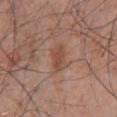Imaged during a routine full-body skin examination; the lesion was not biopsied and no histopathology is available. Cropped from a total-body skin-imaging series; the visible field is about 15 mm. This is a white-light tile. A male subject, approximately 70 years of age. Automated image analysis of the tile measured a lesion area of about 6 mm² and a symmetry-axis asymmetry near 0.25. And it measured a lesion color around L≈49 a*≈21 b*≈28 in CIELAB and roughly 7 lightness units darker than nearby skin. And it measured a color-variation rating of about 2.5/10 and peripheral color asymmetry of about 1. Measured at roughly 4 mm in maximum diameter. Located on the chest.